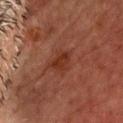subject = male, approximately 65 years of age
illumination = cross-polarized illumination
image = ~15 mm crop, total-body skin-cancer survey
body site = the back
TBP lesion metrics = a within-lesion color-variation index near 2/10 and peripheral color asymmetry of about 0.5; a nevus-likeness score of about 35/100 and a detector confidence of about 100 out of 100 that the crop contains a lesion
lesion size = ≈3 mm